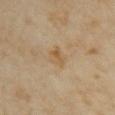Part of a total-body skin-imaging series; this lesion was reviewed on a skin check and was not flagged for biopsy.
A lesion tile, about 15 mm wide, cut from a 3D total-body photograph.
Measured at roughly 2.5 mm in maximum diameter.
The total-body-photography lesion software estimated a lesion area of about 3.5 mm² and an eccentricity of roughly 0.7. And it measured a nevus-likeness score of about 5/100 and a lesion-detection confidence of about 100/100.
A female subject, roughly 35 years of age.
Located on the right upper arm.
Captured under cross-polarized illumination.